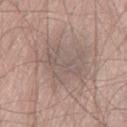Captured during whole-body skin photography for melanoma surveillance; the lesion was not biopsied. A 15 mm crop from a total-body photograph taken for skin-cancer surveillance. The tile uses white-light illumination. A male patient, aged 58–62. The lesion is located on the right thigh. The lesion-visualizer software estimated a lesion area of about 20 mm², an outline eccentricity of about 0.7 (0 = round, 1 = elongated), and two-axis asymmetry of about 0.45. And it measured a border-irregularity rating of about 5.5/10, a within-lesion color-variation index near 2.5/10, and peripheral color asymmetry of about 1. The analysis additionally found a nevus-likeness score of about 0/100.